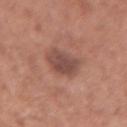| feature | finding |
|---|---|
| follow-up | catalogued during a skin exam; not biopsied |
| acquisition | total-body-photography crop, ~15 mm field of view |
| subject | male, aged around 50 |
| body site | the left forearm |
| illumination | white-light |